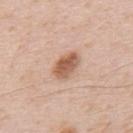Clinical impression:
The lesion was photographed on a routine skin check and not biopsied; there is no pathology result.
Context:
A 15 mm crop from a total-body photograph taken for skin-cancer surveillance. A male subject, approximately 50 years of age. Imaged with white-light lighting. An algorithmic analysis of the crop reported a lesion color around L≈59 a*≈21 b*≈31 in CIELAB and a normalized lesion–skin contrast near 8.5. On the upper back. Longest diameter approximately 4 mm.